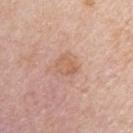Part of a total-body skin-imaging series; this lesion was reviewed on a skin check and was not flagged for biopsy.
A 15 mm close-up extracted from a 3D total-body photography capture.
About 2.5 mm across.
The total-body-photography lesion software estimated a lesion color around L≈61 a*≈21 b*≈31 in CIELAB. It also reported a border-irregularity rating of about 2/10 and a within-lesion color-variation index near 3/10. The software also gave a nevus-likeness score of about 0/100.
This is a white-light tile.
The lesion is on the arm.
A female subject aged approximately 45.